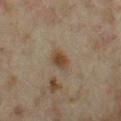The lesion was tiled from a total-body skin photograph and was not biopsied.
Located on the left thigh.
Longest diameter approximately 2.5 mm.
A region of skin cropped from a whole-body photographic capture, roughly 15 mm wide.
A female patient, in their mid-30s.
The total-body-photography lesion software estimated a symmetry-axis asymmetry near 0.15.
Imaged with cross-polarized lighting.